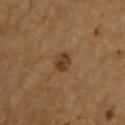<record>
  <biopsy_status>not biopsied; imaged during a skin examination</biopsy_status>
  <site>upper back</site>
  <lighting>cross-polarized</lighting>
  <patient>
    <sex>male</sex>
    <age_approx>85+</age_approx>
  </patient>
  <automated_metrics>
    <area_mm2_approx>4.5</area_mm2_approx>
    <shape_asymmetry>0.15</shape_asymmetry>
  </automated_metrics>
  <lesion_size>
    <long_diameter_mm_approx>2.5</long_diameter_mm_approx>
  </lesion_size>
  <image>
    <source>total-body photography crop</source>
    <field_of_view_mm>15</field_of_view_mm>
  </image>
</record>